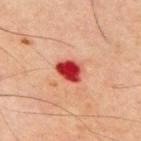follow-up: total-body-photography surveillance lesion; no biopsy | imaging modality: ~15 mm crop, total-body skin-cancer survey | subject: male, in their 70s | diameter: ≈3.5 mm | site: the chest | automated metrics: a footprint of about 6.5 mm², an outline eccentricity of about 0.65 (0 = round, 1 = elongated), and a shape-asymmetry score of about 0.2 (0 = symmetric) | lighting: cross-polarized illumination.